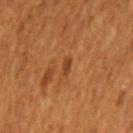notes: no biopsy performed (imaged during a skin exam)
image source: ~15 mm crop, total-body skin-cancer survey
location: the right upper arm
subject: female, aged approximately 50